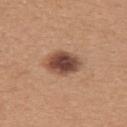| key | value |
|---|---|
| workup | total-body-photography surveillance lesion; no biopsy |
| site | the upper back |
| imaging modality | ~15 mm tile from a whole-body skin photo |
| patient | female, approximately 30 years of age |